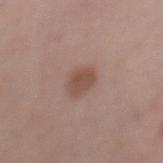{"biopsy_status": "not biopsied; imaged during a skin examination", "patient": {"sex": "female", "age_approx": 50}, "lighting": "white-light", "lesion_size": {"long_diameter_mm_approx": 3.0}, "site": "left thigh", "image": {"source": "total-body photography crop", "field_of_view_mm": 15}}